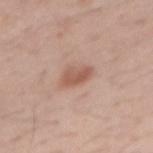Q: Is there a histopathology result?
A: imaged on a skin check; not biopsied
Q: Lesion size?
A: ~3.5 mm (longest diameter)
Q: What are the patient's age and sex?
A: male, aged around 40
Q: Lesion location?
A: the right forearm
Q: What kind of image is this?
A: total-body-photography crop, ~15 mm field of view
Q: What lighting was used for the tile?
A: white-light illumination
Q: Automated lesion metrics?
A: a lesion color around L≈56 a*≈21 b*≈28 in CIELAB, about 10 CIELAB-L* units darker than the surrounding skin, and a normalized border contrast of about 7; a nevus-likeness score of about 60/100 and a lesion-detection confidence of about 100/100